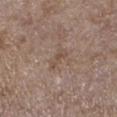Q: Was this lesion biopsied?
A: catalogued during a skin exam; not biopsied
Q: What kind of image is this?
A: ~15 mm crop, total-body skin-cancer survey
Q: Patient demographics?
A: female, roughly 65 years of age
Q: Where on the body is the lesion?
A: the left lower leg